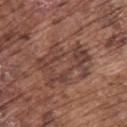workup: total-body-photography surveillance lesion; no biopsy | location: the upper back | patient: male, approximately 75 years of age | image: ~15 mm crop, total-body skin-cancer survey.